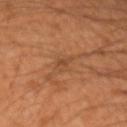The lesion was tiled from a total-body skin photograph and was not biopsied.
A male patient, in their 50s.
A 15 mm close-up tile from a total-body photography series done for melanoma screening.
The recorded lesion diameter is about 2.5 mm.
The lesion is on the left forearm.
An algorithmic analysis of the crop reported an area of roughly 2.5 mm², an outline eccentricity of about 0.9 (0 = round, 1 = elongated), and a symmetry-axis asymmetry near 0.35.